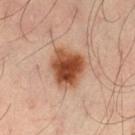No biopsy was performed on this lesion — it was imaged during a full skin examination and was not determined to be concerning.
A male patient, aged around 35.
A 15 mm close-up tile from a total-body photography series done for melanoma screening.
The lesion is located on the left thigh.